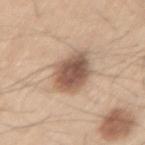The lesion was photographed on a routine skin check and not biopsied; there is no pathology result. A 15 mm close-up extracted from a 3D total-body photography capture. A male patient, aged 58–62. Located on the left thigh.